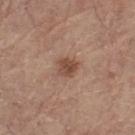notes — catalogued during a skin exam; not biopsied | location — the leg | subject — male, aged around 65 | acquisition — 15 mm crop, total-body photography.